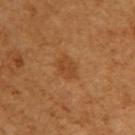follow-up = no biopsy performed (imaged during a skin exam) | subject = female, aged 53–57 | imaging modality = total-body-photography crop, ~15 mm field of view | location = the arm | tile lighting = cross-polarized.